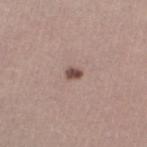Q: What is the imaging modality?
A: ~15 mm tile from a whole-body skin photo
Q: What lighting was used for the tile?
A: white-light illumination
Q: What did automated image analysis measure?
A: an area of roughly 2.5 mm², an eccentricity of roughly 0.65, and a shape-asymmetry score of about 0.25 (0 = symmetric); a lesion-to-skin contrast of about 10 (normalized; higher = more distinct); a color-variation rating of about 2/10 and radial color variation of about 0.5
Q: What are the patient's age and sex?
A: female, aged 23–27
Q: Where on the body is the lesion?
A: the left lower leg
Q: What is the lesion's diameter?
A: ≈2 mm